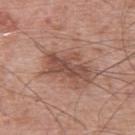Captured during whole-body skin photography for melanoma surveillance; the lesion was not biopsied.
A roughly 15 mm field-of-view crop from a total-body skin photograph.
Approximately 5 mm at its widest.
Imaged with white-light lighting.
A male subject, in their mid- to late 60s.
On the upper back.